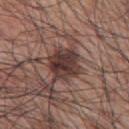Q: Was a biopsy performed?
A: imaged on a skin check; not biopsied
Q: Lesion location?
A: the upper back
Q: How was the tile lit?
A: white-light
Q: How large is the lesion?
A: about 4 mm
Q: What did automated image analysis measure?
A: a nevus-likeness score of about 35/100 and a detector confidence of about 100 out of 100 that the crop contains a lesion
Q: What kind of image is this?
A: 15 mm crop, total-body photography
Q: Patient demographics?
A: male, aged approximately 70